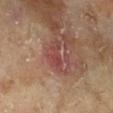Recorded during total-body skin imaging; not selected for excision or biopsy.
A 15 mm close-up tile from a total-body photography series done for melanoma screening.
The subject is a male in their mid- to late 60s.
This is a cross-polarized tile.
From the right lower leg.
The lesion's longest dimension is about 3.5 mm.